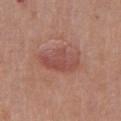Imaged during a routine full-body skin examination; the lesion was not biopsied and no histopathology is available.
The patient is a female aged around 65.
On the chest.
The recorded lesion diameter is about 6 mm.
Automated image analysis of the tile measured a footprint of about 13 mm², a shape eccentricity near 0.9, and two-axis asymmetry of about 0.25. And it measured a border-irregularity rating of about 3/10, internal color variation of about 3 on a 0–10 scale, and peripheral color asymmetry of about 1.
This image is a 15 mm lesion crop taken from a total-body photograph.
The tile uses white-light illumination.